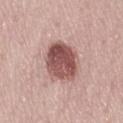{
  "biopsy_status": "not biopsied; imaged during a skin examination",
  "lesion_size": {
    "long_diameter_mm_approx": 6.0
  },
  "lighting": "white-light",
  "image": {
    "source": "total-body photography crop",
    "field_of_view_mm": 15
  },
  "site": "lower back",
  "patient": {
    "sex": "male",
    "age_approx": 70
  }
}